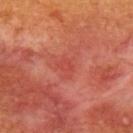{"biopsy_status": "not biopsied; imaged during a skin examination", "patient": {"sex": "female", "age_approx": 55}, "image": {"source": "total-body photography crop", "field_of_view_mm": 15}, "site": "back"}